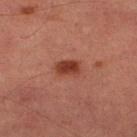Impression:
Part of a total-body skin-imaging series; this lesion was reviewed on a skin check and was not flagged for biopsy.
Clinical summary:
On the right lower leg. The subject is a male in their mid- to late 80s or older. Cropped from a whole-body photographic skin survey; the tile spans about 15 mm. This is a cross-polarized tile.